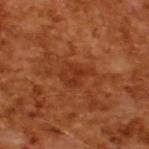follow-up = imaged on a skin check; not biopsied
image source = ~15 mm tile from a whole-body skin photo
subject = male, aged 63–67
lighting = cross-polarized illumination
size = ~3.5 mm (longest diameter)
automated metrics = a lesion color around L≈34 a*≈28 b*≈35 in CIELAB, about 6 CIELAB-L* units darker than the surrounding skin, and a normalized lesion–skin contrast near 6